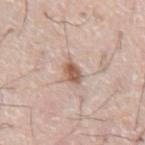follow-up: catalogued during a skin exam; not biopsied
anatomic site: the front of the torso
diameter: about 3 mm
image-analysis metrics: a footprint of about 4.5 mm², a shape eccentricity near 0.75, and a shape-asymmetry score of about 0.25 (0 = symmetric); a lesion–skin lightness drop of about 13; a nevus-likeness score of about 90/100
image source: ~15 mm crop, total-body skin-cancer survey
subject: male, in their mid-70s